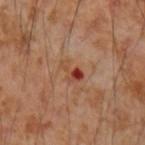Findings:
• follow-up · total-body-photography surveillance lesion; no biopsy
• size · ≈3 mm
• image · ~15 mm tile from a whole-body skin photo
• subject · male, approximately 55 years of age
• body site · the right forearm